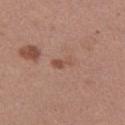Assessment: Part of a total-body skin-imaging series; this lesion was reviewed on a skin check and was not flagged for biopsy. Image and clinical context: A female patient about 35 years old. Approximately 2.5 mm at its widest. A 15 mm close-up tile from a total-body photography series done for melanoma screening. Located on the right thigh.